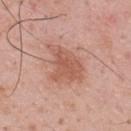Impression: No biopsy was performed on this lesion — it was imaged during a full skin examination and was not determined to be concerning. Image and clinical context: The recorded lesion diameter is about 5 mm. The lesion is on the upper back. The patient is a male aged around 55. A roughly 15 mm field-of-view crop from a total-body skin photograph. This is a white-light tile.